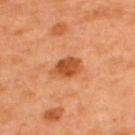Impression: The lesion was photographed on a routine skin check and not biopsied; there is no pathology result. Image and clinical context: On the upper back. Measured at roughly 4 mm in maximum diameter. A male patient, approximately 50 years of age. A 15 mm crop from a total-body photograph taken for skin-cancer surveillance.